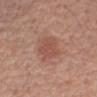{
  "biopsy_status": "not biopsied; imaged during a skin examination",
  "image": {
    "source": "total-body photography crop",
    "field_of_view_mm": 15
  },
  "lighting": "white-light",
  "automated_metrics": {
    "border_irregularity_0_10": 3.0,
    "color_variation_0_10": 2.5,
    "peripheral_color_asymmetry": 1.0
  },
  "patient": {
    "sex": "male",
    "age_approx": 60
  },
  "site": "right upper arm"
}